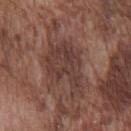Notes:
- follow-up: imaged on a skin check; not biopsied
- body site: the chest
- subject: male, in their mid- to late 70s
- diameter: about 6.5 mm
- tile lighting: white-light
- acquisition: 15 mm crop, total-body photography